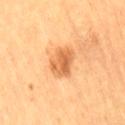imaging modality = total-body-photography crop, ~15 mm field of view
illumination = cross-polarized
location = the right thigh
lesion size = ~3.5 mm (longest diameter)
image-analysis metrics = a footprint of about 8 mm² and a shape eccentricity near 0.65; a mean CIELAB color near L≈63 a*≈27 b*≈44 and a normalized border contrast of about 7.5; a color-variation rating of about 4.5/10 and a peripheral color-asymmetry measure near 1.5; a classifier nevus-likeness of about 70/100 and a detector confidence of about 100 out of 100 that the crop contains a lesion
subject = female, about 70 years old